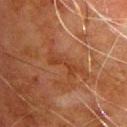Imaged with cross-polarized lighting. The total-body-photography lesion software estimated a footprint of about 5 mm², a shape eccentricity near 0.95, and two-axis asymmetry of about 0.5. And it measured a border-irregularity rating of about 7.5/10, a within-lesion color-variation index near 0.5/10, and radial color variation of about 0. It also reported a nevus-likeness score of about 0/100 and a detector confidence of about 90 out of 100 that the crop contains a lesion. A male subject roughly 80 years of age. The lesion is located on the chest. A close-up tile cropped from a whole-body skin photograph, about 15 mm across.